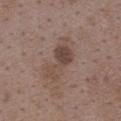The lesion was photographed on a routine skin check and not biopsied; there is no pathology result. The tile uses white-light illumination. A 15 mm close-up tile from a total-body photography series done for melanoma screening. The lesion is on the upper back. A female patient, in their mid-30s. The lesion-visualizer software estimated an area of roughly 12 mm², an eccentricity of roughly 0.9, and two-axis asymmetry of about 0.3. The analysis additionally found a classifier nevus-likeness of about 5/100 and lesion-presence confidence of about 100/100.